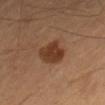The lesion was tiled from a total-body skin photograph and was not biopsied. The patient is a male roughly 60 years of age. From the right thigh. The lesion's longest dimension is about 3.5 mm. Cropped from a total-body skin-imaging series; the visible field is about 15 mm.